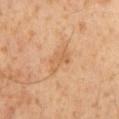follow-up = total-body-photography surveillance lesion; no biopsy | site = the mid back | illumination = cross-polarized | image = ~15 mm crop, total-body skin-cancer survey | subject = male, aged 58–62 | lesion size = ~4 mm (longest diameter).